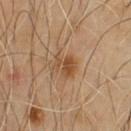No biopsy was performed on this lesion — it was imaged during a full skin examination and was not determined to be concerning. A 15 mm crop from a total-body photograph taken for skin-cancer surveillance. The total-body-photography lesion software estimated a footprint of about 5.5 mm² and a shape-asymmetry score of about 0.3 (0 = symmetric). The analysis additionally found about 9 CIELAB-L* units darker than the surrounding skin. The analysis additionally found a border-irregularity rating of about 4.5/10, a within-lesion color-variation index near 2.5/10, and a peripheral color-asymmetry measure near 1. It also reported an automated nevus-likeness rating near 80 out of 100. The patient is a male aged approximately 55. Located on the chest. This is a cross-polarized tile.